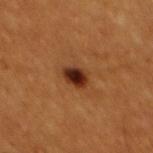{"biopsy_status": "not biopsied; imaged during a skin examination", "lighting": "cross-polarized", "automated_metrics": {"area_mm2_approx": 5.5, "eccentricity": 0.45, "shape_asymmetry": 0.2}, "image": {"source": "total-body photography crop", "field_of_view_mm": 15}, "patient": {"sex": "male", "age_approx": 60}, "site": "mid back", "lesion_size": {"long_diameter_mm_approx": 3.0}}